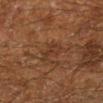biopsy status: total-body-photography surveillance lesion; no biopsy | body site: the left lower leg | acquisition: 15 mm crop, total-body photography | subject: male, about 60 years old | diameter: ~3 mm (longest diameter) | lighting: cross-polarized.